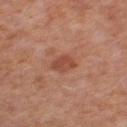Q: Was this lesion biopsied?
A: catalogued during a skin exam; not biopsied
Q: What is the imaging modality?
A: total-body-photography crop, ~15 mm field of view
Q: Who is the patient?
A: female, aged 48 to 52
Q: Lesion location?
A: the leg